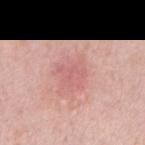This lesion was catalogued during total-body skin photography and was not selected for biopsy. A roughly 15 mm field-of-view crop from a total-body skin photograph. Located on the arm. Automated image analysis of the tile measured a normalized border contrast of about 3. The subject is a male about 40 years old.